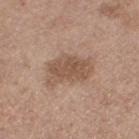Impression: This lesion was catalogued during total-body skin photography and was not selected for biopsy. Context: Imaged with white-light lighting. A female patient, in their mid- to late 60s. About 5.5 mm across. An algorithmic analysis of the crop reported a footprint of about 12 mm², an outline eccentricity of about 0.8 (0 = round, 1 = elongated), and two-axis asymmetry of about 0.3. The analysis additionally found a nevus-likeness score of about 10/100 and a detector confidence of about 100 out of 100 that the crop contains a lesion. A region of skin cropped from a whole-body photographic capture, roughly 15 mm wide. Located on the left thigh.